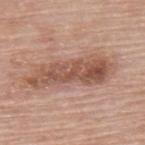The lesion was photographed on a routine skin check and not biopsied; there is no pathology result.
Cropped from a whole-body photographic skin survey; the tile spans about 15 mm.
A male patient, about 80 years old.
The lesion is on the upper back.